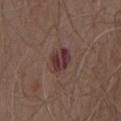{"biopsy_status": "not biopsied; imaged during a skin examination", "patient": {"sex": "male", "age_approx": 70}, "image": {"source": "total-body photography crop", "field_of_view_mm": 15}, "site": "chest", "lesion_size": {"long_diameter_mm_approx": 3.0}, "lighting": "white-light", "automated_metrics": {"cielab_L": 34, "cielab_a": 21, "cielab_b": 18, "vs_skin_darker_L": 10.0, "vs_skin_contrast_norm": 10.0}}